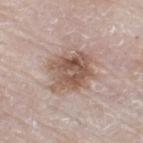Impression:
The lesion was photographed on a routine skin check and not biopsied; there is no pathology result.
Acquisition and patient details:
Cropped from a whole-body photographic skin survey; the tile spans about 15 mm. The recorded lesion diameter is about 5 mm. A male patient, about 80 years old. Imaged with white-light lighting. On the right thigh.